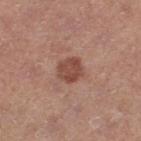Assessment:
The lesion was tiled from a total-body skin photograph and was not biopsied.
Image and clinical context:
A 15 mm crop from a total-body photograph taken for skin-cancer surveillance. Automated image analysis of the tile measured an average lesion color of about L≈47 a*≈23 b*≈27 (CIELAB) and a lesion-to-skin contrast of about 8 (normalized; higher = more distinct). The analysis additionally found a border-irregularity rating of about 2/10 and a peripheral color-asymmetry measure near 1. From the left thigh. Captured under white-light illumination. A female subject, aged around 40.